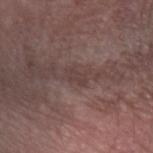Recorded during total-body skin imaging; not selected for excision or biopsy.